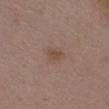follow-up: no biopsy performed (imaged during a skin exam); patient: female, about 35 years old; image source: total-body-photography crop, ~15 mm field of view; lesion size: about 2.5 mm; TBP lesion metrics: border irregularity of about 3 on a 0–10 scale, internal color variation of about 1.5 on a 0–10 scale, and peripheral color asymmetry of about 0.5; site: the chest; tile lighting: white-light.